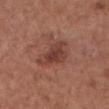Findings:
• notes: total-body-photography surveillance lesion; no biopsy
• image: ~15 mm crop, total-body skin-cancer survey
• location: the chest
• subject: female, aged 73–77
• lesion size: ~4.5 mm (longest diameter)
• lighting: white-light
• image-analysis metrics: a mean CIELAB color near L≈41 a*≈24 b*≈25; a within-lesion color-variation index near 3.5/10 and radial color variation of about 1; a classifier nevus-likeness of about 35/100